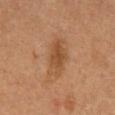notes = no biopsy performed (imaged during a skin exam)
patient = male, about 60 years old
imaging modality = total-body-photography crop, ~15 mm field of view
tile lighting = cross-polarized
TBP lesion metrics = a lesion area of about 10 mm² and a shape eccentricity near 0.9; a border-irregularity index near 3.5/10 and a within-lesion color-variation index near 3/10; a nevus-likeness score of about 50/100 and lesion-presence confidence of about 100/100
lesion diameter = about 5 mm
site = the mid back